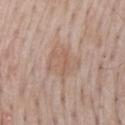Clinical impression: Part of a total-body skin-imaging series; this lesion was reviewed on a skin check and was not flagged for biopsy. Context: An algorithmic analysis of the crop reported a footprint of about 12 mm², an outline eccentricity of about 0.5 (0 = round, 1 = elongated), and a shape-asymmetry score of about 0.3 (0 = symmetric). The analysis additionally found a lesion color around L≈59 a*≈17 b*≈27 in CIELAB, a lesion–skin lightness drop of about 6, and a normalized border contrast of about 4.5. It also reported a border-irregularity rating of about 3.5/10 and internal color variation of about 3 on a 0–10 scale. Cropped from a total-body skin-imaging series; the visible field is about 15 mm. The lesion is located on the mid back. This is a white-light tile. A male subject, aged approximately 65.